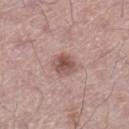Q: Was a biopsy performed?
A: no biopsy performed (imaged during a skin exam)
Q: Where on the body is the lesion?
A: the leg
Q: What kind of image is this?
A: ~15 mm crop, total-body skin-cancer survey
Q: How was the tile lit?
A: white-light illumination
Q: What did automated image analysis measure?
A: an automated nevus-likeness rating near 75 out of 100 and a lesion-detection confidence of about 100/100
Q: Patient demographics?
A: male, about 50 years old